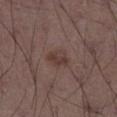<record>
  <biopsy_status>not biopsied; imaged during a skin examination</biopsy_status>
  <site>right thigh</site>
  <lighting>white-light</lighting>
  <patient>
    <sex>male</sex>
    <age_approx>50</age_approx>
  </patient>
  <automated_metrics>
    <eccentricity>0.65</eccentricity>
    <shape_asymmetry>0.25</shape_asymmetry>
    <color_variation_0_10>3.0</color_variation_0_10>
    <peripheral_color_asymmetry>1.0</peripheral_color_asymmetry>
    <nevus_likeness_0_100>5</nevus_likeness_0_100>
    <lesion_detection_confidence_0_100>100</lesion_detection_confidence_0_100>
  </automated_metrics>
  <image>
    <source>total-body photography crop</source>
    <field_of_view_mm>15</field_of_view_mm>
  </image>
</record>